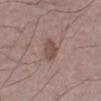{"biopsy_status": "not biopsied; imaged during a skin examination", "site": "left thigh", "lighting": "white-light", "image": {"source": "total-body photography crop", "field_of_view_mm": 15}, "patient": {"sex": "male", "age_approx": 50}, "automated_metrics": {"area_mm2_approx": 5.5, "eccentricity": 0.4, "shape_asymmetry": 0.25, "border_irregularity_0_10": 2.5, "color_variation_0_10": 2.0, "peripheral_color_asymmetry": 0.5, "lesion_detection_confidence_0_100": 100}, "lesion_size": {"long_diameter_mm_approx": 2.5}}